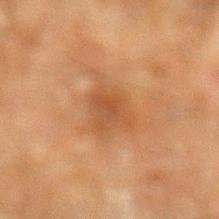No biopsy was performed on this lesion — it was imaged during a full skin examination and was not determined to be concerning. A male subject, approximately 60 years of age. From the left lower leg. A region of skin cropped from a whole-body photographic capture, roughly 15 mm wide. Captured under cross-polarized illumination. Longest diameter approximately 5 mm.